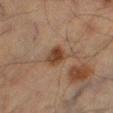Captured during whole-body skin photography for melanoma surveillance; the lesion was not biopsied.
A roughly 15 mm field-of-view crop from a total-body skin photograph.
Longest diameter approximately 3 mm.
The lesion is on the left thigh.
The tile uses cross-polarized illumination.
A male subject, roughly 65 years of age.